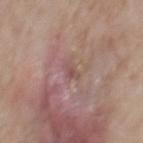Recorded during total-body skin imaging; not selected for excision or biopsy. The patient is a male aged 78 to 82. This is a white-light tile. Longest diameter approximately 2.5 mm. The lesion is on the front of the torso. A close-up tile cropped from a whole-body skin photograph, about 15 mm across.